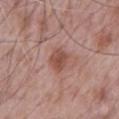workup: imaged on a skin check; not biopsied | diameter: ~2.5 mm (longest diameter) | lighting: white-light illumination | subject: male, aged 68 to 72 | anatomic site: the chest | imaging modality: 15 mm crop, total-body photography.